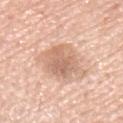Assessment: Recorded during total-body skin imaging; not selected for excision or biopsy. Image and clinical context: A close-up tile cropped from a whole-body skin photograph, about 15 mm across. About 4.5 mm across. A male patient, approximately 65 years of age. This is a white-light tile. From the upper back.